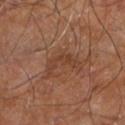notes=no biopsy performed (imaged during a skin exam).